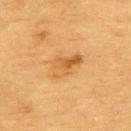{"biopsy_status": "not biopsied; imaged during a skin examination", "patient": {"sex": "male", "age_approx": "85+"}, "site": "upper back", "image": {"source": "total-body photography crop", "field_of_view_mm": 15}, "lesion_size": {"long_diameter_mm_approx": 4.5}, "automated_metrics": {"eccentricity": 0.8, "shape_asymmetry": 0.35, "cielab_L": 52, "cielab_a": 20, "cielab_b": 42, "vs_skin_darker_L": 8.0, "vs_skin_contrast_norm": 6.0}, "lighting": "cross-polarized"}